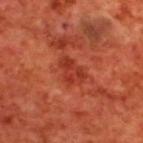* subject: male, about 70 years old
* imaging modality: total-body-photography crop, ~15 mm field of view
* lesion size: about 3.5 mm
* site: the upper back
* automated lesion analysis: an automated nevus-likeness rating near 0 out of 100 and a lesion-detection confidence of about 100/100
* lighting: cross-polarized illumination